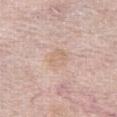- biopsy status: no biopsy performed (imaged during a skin exam)